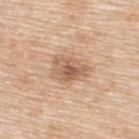Case summary:
• follow-up — imaged on a skin check; not biopsied
• size — ≈4.5 mm
• image source — 15 mm crop, total-body photography
• subject — female, about 55 years old
• image-analysis metrics — an area of roughly 10 mm² and an eccentricity of roughly 0.7
• location — the upper back
• lighting — white-light